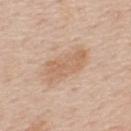- workup — no biopsy performed (imaged during a skin exam)
- body site — the upper back
- image source — 15 mm crop, total-body photography
- diameter — ~5.5 mm (longest diameter)
- tile lighting — white-light illumination
- patient — male, in their 60s